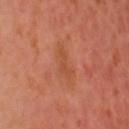biopsy_status: not biopsied; imaged during a skin examination
patient:
  sex: male
  age_approx: 55
lighting: cross-polarized
lesion_size:
  long_diameter_mm_approx: 3.0
site: head or neck
image:
  source: total-body photography crop
  field_of_view_mm: 15